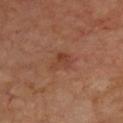No biopsy was performed on this lesion — it was imaged during a full skin examination and was not determined to be concerning. This is a cross-polarized tile. A 15 mm close-up tile from a total-body photography series done for melanoma screening. Automated tile analysis of the lesion measured a border-irregularity rating of about 4/10 and peripheral color asymmetry of about 1. The analysis additionally found a nevus-likeness score of about 15/100 and lesion-presence confidence of about 100/100. The patient is a male aged approximately 65.